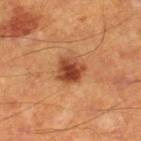Q: Was a biopsy performed?
A: no biopsy performed (imaged during a skin exam)
Q: Where on the body is the lesion?
A: the right lower leg
Q: How was this image acquired?
A: total-body-photography crop, ~15 mm field of view
Q: What are the patient's age and sex?
A: male, aged 58 to 62